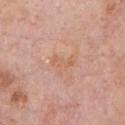The lesion was tiled from a total-body skin photograph and was not biopsied.
A male subject aged around 60.
The lesion is located on the front of the torso.
Automated tile analysis of the lesion measured a mean CIELAB color near L≈61 a*≈22 b*≈32 and a normalized border contrast of about 5. The software also gave a within-lesion color-variation index near 0/10. And it measured a detector confidence of about 100 out of 100 that the crop contains a lesion.
A roughly 15 mm field-of-view crop from a total-body skin photograph.
The lesion's longest dimension is about 3 mm.